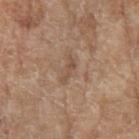| feature | finding |
|---|---|
| notes | no biopsy performed (imaged during a skin exam) |
| acquisition | 15 mm crop, total-body photography |
| patient | female, about 75 years old |
| automated metrics | roughly 8 lightness units darker than nearby skin and a normalized border contrast of about 5.5; border irregularity of about 6.5 on a 0–10 scale and internal color variation of about 0 on a 0–10 scale; an automated nevus-likeness rating near 0 out of 100 and lesion-presence confidence of about 95/100 |
| body site | the right forearm |
| lighting | white-light |